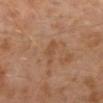{"biopsy_status": "not biopsied; imaged during a skin examination", "image": {"source": "total-body photography crop", "field_of_view_mm": 15}, "lesion_size": {"long_diameter_mm_approx": 3.0}, "patient": {"sex": "male", "age_approx": 30}, "lighting": "cross-polarized", "site": "left lower leg"}